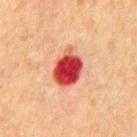biopsy status = imaged on a skin check; not biopsied.